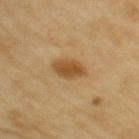biopsy_status: not biopsied; imaged during a skin examination
patient:
  sex: female
  age_approx: 70
automated_metrics:
  cielab_L: 52
  cielab_a: 19
  cielab_b: 41
  vs_skin_darker_L: 11.0
  vs_skin_contrast_norm: 8.5
  border_irregularity_0_10: 2.0
  color_variation_0_10: 2.5
  nevus_likeness_0_100: 95
lighting: cross-polarized
lesion_size:
  long_diameter_mm_approx: 4.5
image:
  source: total-body photography crop
  field_of_view_mm: 15
site: right upper arm